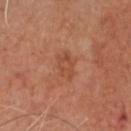{"biopsy_status": "not biopsied; imaged during a skin examination"}